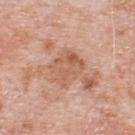Clinical impression:
Imaged during a routine full-body skin examination; the lesion was not biopsied and no histopathology is available.
Acquisition and patient details:
A male subject, aged 78 to 82. This is a white-light tile. A roughly 15 mm field-of-view crop from a total-body skin photograph. From the upper back. Approximately 4 mm at its widest.